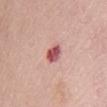No biopsy was performed on this lesion — it was imaged during a full skin examination and was not determined to be concerning.
A lesion tile, about 15 mm wide, cut from a 3D total-body photograph.
The lesion is located on the abdomen.
A female patient roughly 65 years of age.
An algorithmic analysis of the crop reported a lesion-to-skin contrast of about 10.5 (normalized; higher = more distinct). It also reported an automated nevus-likeness rating near 10 out of 100 and a lesion-detection confidence of about 100/100.